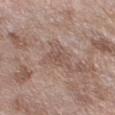biopsy status: total-body-photography surveillance lesion; no biopsy | lesion size: ~4 mm (longest diameter) | image: 15 mm crop, total-body photography | subject: male, approximately 70 years of age | body site: the right forearm.